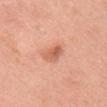biopsy status = catalogued during a skin exam; not biopsied | site = the head or neck | illumination = white-light illumination | imaging modality = total-body-photography crop, ~15 mm field of view | patient = female, in their mid-30s | size = about 3 mm.